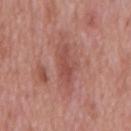Findings:
– biopsy status · no biopsy performed (imaged during a skin exam)
– subject · male, about 65 years old
– anatomic site · the mid back
– lesion size · about 4 mm
– imaging modality · ~15 mm crop, total-body skin-cancer survey